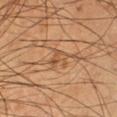<case>
<biopsy_status>not biopsied; imaged during a skin examination</biopsy_status>
<lighting>cross-polarized</lighting>
<image>
  <source>total-body photography crop</source>
  <field_of_view_mm>15</field_of_view_mm>
</image>
<automated_metrics>
  <area_mm2_approx>4.0</area_mm2_approx>
  <cielab_L>52</cielab_L>
  <cielab_a>21</cielab_a>
  <cielab_b>36</cielab_b>
  <vs_skin_contrast_norm>5.5</vs_skin_contrast_norm>
</automated_metrics>
<patient>
  <sex>male</sex>
  <age_approx>60</age_approx>
</patient>
<lesion_size>
  <long_diameter_mm_approx>3.0</long_diameter_mm_approx>
</lesion_size>
<site>right lower leg</site>
</case>